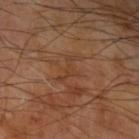Q: How large is the lesion?
A: about 4 mm
Q: Where on the body is the lesion?
A: the left upper arm
Q: What kind of image is this?
A: ~15 mm tile from a whole-body skin photo
Q: Patient demographics?
A: male, aged approximately 65
Q: Automated lesion metrics?
A: a footprint of about 4.5 mm² and two-axis asymmetry of about 0.8; an average lesion color of about L≈40 a*≈21 b*≈31 (CIELAB) and a normalized lesion–skin contrast near 5; a border-irregularity index near 9.5/10 and radial color variation of about 0; a nevus-likeness score of about 0/100 and a lesion-detection confidence of about 85/100